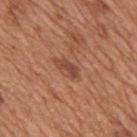Imaged during a routine full-body skin examination; the lesion was not biopsied and no histopathology is available.
Cropped from a whole-body photographic skin survey; the tile spans about 15 mm.
Located on the mid back.
A male subject roughly 65 years of age.